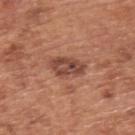Background: The lesion is on the upper back. A male subject aged 63 to 67. A 15 mm close-up extracted from a 3D total-body photography capture.